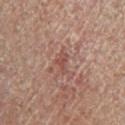{
  "biopsy_status": "not biopsied; imaged during a skin examination",
  "site": "left lower leg",
  "image": {
    "source": "total-body photography crop",
    "field_of_view_mm": 15
  },
  "patient": {
    "sex": "male",
    "age_approx": 70
  }
}